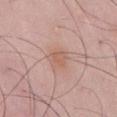Q: Was this lesion biopsied?
A: no biopsy performed (imaged during a skin exam)
Q: What is the lesion's diameter?
A: ~3 mm (longest diameter)
Q: How was this image acquired?
A: total-body-photography crop, ~15 mm field of view
Q: Automated lesion metrics?
A: a shape eccentricity near 0.6 and a symmetry-axis asymmetry near 0.2; a border-irregularity index near 2/10, a color-variation rating of about 2/10, and radial color variation of about 0.5; a nevus-likeness score of about 0/100 and a detector confidence of about 100 out of 100 that the crop contains a lesion
Q: Illumination type?
A: white-light illumination
Q: Patient demographics?
A: male, aged approximately 50
Q: What is the anatomic site?
A: the chest